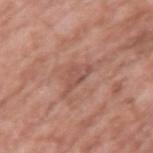No biopsy was performed on this lesion — it was imaged during a full skin examination and was not determined to be concerning. The subject is a male aged 78 to 82. Located on the left upper arm. The recorded lesion diameter is about 4 mm. A close-up tile cropped from a whole-body skin photograph, about 15 mm across.